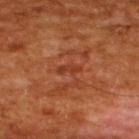Q: Is there a histopathology result?
A: no biopsy performed (imaged during a skin exam)
Q: What are the patient's age and sex?
A: male, aged 63–67
Q: What kind of image is this?
A: ~15 mm tile from a whole-body skin photo
Q: What lighting was used for the tile?
A: cross-polarized
Q: Where on the body is the lesion?
A: the upper back
Q: Lesion size?
A: ≈2.5 mm